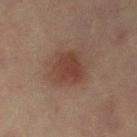Assessment: Recorded during total-body skin imaging; not selected for excision or biopsy. Context: A close-up tile cropped from a whole-body skin photograph, about 15 mm across. The recorded lesion diameter is about 4 mm. This is a cross-polarized tile. From the left lower leg. The subject is a female approximately 55 years of age.